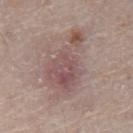  biopsy_status: not biopsied; imaged during a skin examination
  lesion_size:
    long_diameter_mm_approx: 7.5
  site: chest
  image:
    source: total-body photography crop
    field_of_view_mm: 15
  automated_metrics:
    area_mm2_approx: 19.0
    eccentricity: 0.85
    vs_skin_darker_L: 10.0
    vs_skin_contrast_norm: 7.0
    nevus_likeness_0_100: 10
    lesion_detection_confidence_0_100: 95
  patient:
    sex: male
    age_approx: 80